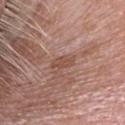The lesion was photographed on a routine skin check and not biopsied; there is no pathology result.
Cropped from a whole-body photographic skin survey; the tile spans about 15 mm.
On the head or neck.
A male subject, approximately 50 years of age.